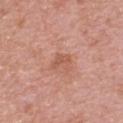Q: Is there a histopathology result?
A: imaged on a skin check; not biopsied
Q: Patient demographics?
A: female, aged 63 to 67
Q: What kind of image is this?
A: ~15 mm tile from a whole-body skin photo
Q: Lesion location?
A: the upper back